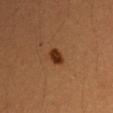Notes:
– workup: imaged on a skin check; not biopsied
– image source: total-body-photography crop, ~15 mm field of view
– subject: female, aged 38–42
– anatomic site: the left upper arm